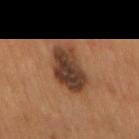No biopsy was performed on this lesion — it was imaged during a full skin examination and was not determined to be concerning.
From the mid back.
Imaged with cross-polarized lighting.
A male subject in their mid-50s.
Cropped from a total-body skin-imaging series; the visible field is about 15 mm.
Measured at roughly 6 mm in maximum diameter.